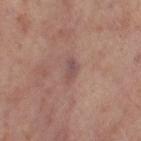{"biopsy_status": "not biopsied; imaged during a skin examination", "lighting": "cross-polarized", "image": {"source": "total-body photography crop", "field_of_view_mm": 15}, "patient": {"sex": "female", "age_approx": 55}, "site": "right thigh", "lesion_size": {"long_diameter_mm_approx": 3.0}}